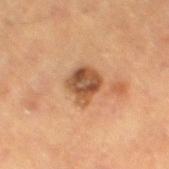This lesion was catalogued during total-body skin photography and was not selected for biopsy. A 15 mm close-up extracted from a 3D total-body photography capture. A male patient aged 83 or older. Automated image analysis of the tile measured a mean CIELAB color near L≈44 a*≈20 b*≈31, about 13 CIELAB-L* units darker than the surrounding skin, and a normalized border contrast of about 9.5. The analysis additionally found a border-irregularity index near 2/10, internal color variation of about 5.5 on a 0–10 scale, and radial color variation of about 2. On the left thigh. This is a cross-polarized tile.